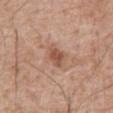Q: Was this lesion biopsied?
A: imaged on a skin check; not biopsied
Q: What is the lesion's diameter?
A: ~3 mm (longest diameter)
Q: Lesion location?
A: the abdomen
Q: Who is the patient?
A: male, approximately 60 years of age
Q: What kind of image is this?
A: total-body-photography crop, ~15 mm field of view